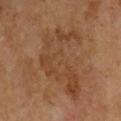No biopsy was performed on this lesion — it was imaged during a full skin examination and was not determined to be concerning. Cropped from a total-body skin-imaging series; the visible field is about 15 mm. Located on the left arm. A male subject, aged 63 to 67. Captured under cross-polarized illumination.